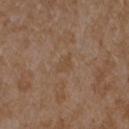The lesion was tiled from a total-body skin photograph and was not biopsied.
A female subject roughly 35 years of age.
An algorithmic analysis of the crop reported an area of roughly 3.5 mm². The analysis additionally found an average lesion color of about L≈47 a*≈16 b*≈30 (CIELAB), about 4 CIELAB-L* units darker than the surrounding skin, and a normalized border contrast of about 4.5. And it measured border irregularity of about 4 on a 0–10 scale, a within-lesion color-variation index near 1/10, and radial color variation of about 0.5.
The tile uses white-light illumination.
The lesion is located on the right forearm.
Cropped from a whole-body photographic skin survey; the tile spans about 15 mm.
The recorded lesion diameter is about 2.5 mm.